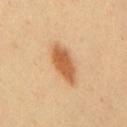Findings:
- biopsy status · catalogued during a skin exam; not biopsied
- patient · male, aged 38–42
- body site · the chest
- image source · ~15 mm tile from a whole-body skin photo
- tile lighting · cross-polarized illumination
- size · ~5 mm (longest diameter)